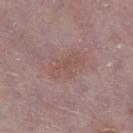Clinical impression:
Recorded during total-body skin imaging; not selected for excision or biopsy.
Image and clinical context:
A male subject aged 73 to 77. On the right lower leg. The recorded lesion diameter is about 4.5 mm. This image is a 15 mm lesion crop taken from a total-body photograph.